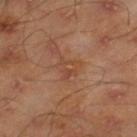Recorded during total-body skin imaging; not selected for excision or biopsy. A 15 mm close-up tile from a total-body photography series done for melanoma screening. The recorded lesion diameter is about 3 mm. From the right lower leg. Automated image analysis of the tile measured a mean CIELAB color near L≈45 a*≈22 b*≈32, roughly 6 lightness units darker than nearby skin, and a normalized lesion–skin contrast near 5. It also reported border irregularity of about 6 on a 0–10 scale, a color-variation rating of about 4.5/10, and radial color variation of about 1.5. The analysis additionally found a lesion-detection confidence of about 100/100. The patient is a male roughly 45 years of age.